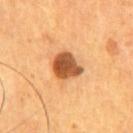body site=the chest
imaging modality=total-body-photography crop, ~15 mm field of view
patient=male, approximately 55 years of age